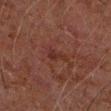workup = catalogued during a skin exam; not biopsied
body site = the arm
patient = male, aged around 60
image = total-body-photography crop, ~15 mm field of view
size = about 2.5 mm